Automated tile analysis of the lesion measured border irregularity of about 9 on a 0–10 scale, a within-lesion color-variation index near 5/10, and radial color variation of about 2. And it measured a detector confidence of about 15 out of 100 that the crop contains a lesion · this image is a 15 mm lesion crop taken from a total-body photograph · imaged with white-light lighting · the recorded lesion diameter is about 10 mm · the patient is a male roughly 65 years of age · the lesion is located on the left lower leg.
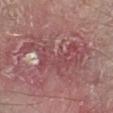pathology: an invasive squamous cell carcinoma, keratoacanthoma type — a malignant skin lesion.